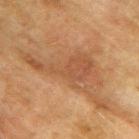This lesion was catalogued during total-body skin photography and was not selected for biopsy. The lesion's longest dimension is about 9.5 mm. On the upper back. Automated tile analysis of the lesion measured a footprint of about 19 mm², a shape eccentricity near 0.9, and two-axis asymmetry of about 0.65. The software also gave an average lesion color of about L≈43 a*≈19 b*≈31 (CIELAB) and a normalized lesion–skin contrast near 6. The analysis additionally found a border-irregularity index near 9/10 and radial color variation of about 1.5. A region of skin cropped from a whole-body photographic capture, roughly 15 mm wide. A male patient aged 73–77. The tile uses cross-polarized illumination.